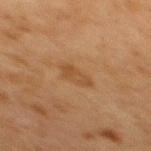follow-up — imaged on a skin check; not biopsied